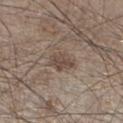An algorithmic analysis of the crop reported a mean CIELAB color near L≈46 a*≈14 b*≈23, roughly 9 lightness units darker than nearby skin, and a lesion-to-skin contrast of about 7 (normalized; higher = more distinct). The analysis additionally found a border-irregularity rating of about 2.5/10, internal color variation of about 2.5 on a 0–10 scale, and peripheral color asymmetry of about 1. And it measured a lesion-detection confidence of about 85/100. A 15 mm crop from a total-body photograph taken for skin-cancer surveillance. The lesion is located on the left lower leg. The lesion's longest dimension is about 3.5 mm. The subject is a male roughly 45 years of age.